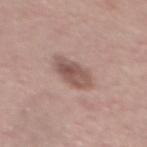The lesion was photographed on a routine skin check and not biopsied; there is no pathology result.
The total-body-photography lesion software estimated an outline eccentricity of about 0.8 (0 = round, 1 = elongated) and a symmetry-axis asymmetry near 0.2. It also reported a mean CIELAB color near L≈53 a*≈19 b*≈22 and a lesion–skin lightness drop of about 11. It also reported a within-lesion color-variation index near 5/10 and peripheral color asymmetry of about 2.
A male subject aged approximately 40.
Approximately 4.5 mm at its widest.
Captured under white-light illumination.
The lesion is on the back.
This image is a 15 mm lesion crop taken from a total-body photograph.